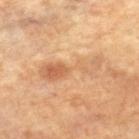Q: Is there a histopathology result?
A: no biopsy performed (imaged during a skin exam)
Q: How was the tile lit?
A: cross-polarized illumination
Q: What kind of image is this?
A: 15 mm crop, total-body photography
Q: What is the lesion's diameter?
A: ≈7 mm
Q: Who is the patient?
A: female, aged 63–67
Q: Where on the body is the lesion?
A: the left leg
Q: What did automated image analysis measure?
A: border irregularity of about 6.5 on a 0–10 scale, internal color variation of about 7 on a 0–10 scale, and a peripheral color-asymmetry measure near 2; a nevus-likeness score of about 0/100